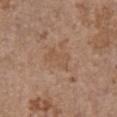{"biopsy_status": "not biopsied; imaged during a skin examination", "site": "chest", "automated_metrics": {"cielab_L": 52, "cielab_a": 18, "cielab_b": 31, "vs_skin_darker_L": 5.0, "vs_skin_contrast_norm": 4.5, "nevus_likeness_0_100": 0, "lesion_detection_confidence_0_100": 100}, "lighting": "white-light", "patient": {"sex": "female", "age_approx": 65}, "lesion_size": {"long_diameter_mm_approx": 3.5}, "image": {"source": "total-body photography crop", "field_of_view_mm": 15}}